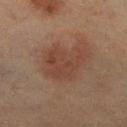Findings:
• biopsy status — imaged on a skin check; not biopsied
• tile lighting — cross-polarized illumination
• location — the right lower leg
• subject — female, approximately 55 years of age
• acquisition — ~15 mm crop, total-body skin-cancer survey